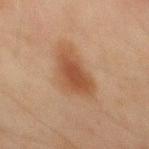| feature | finding |
|---|---|
| biopsy status | imaged on a skin check; not biopsied |
| body site | the mid back |
| diameter | ~6 mm (longest diameter) |
| patient | male, approximately 45 years of age |
| imaging modality | ~15 mm tile from a whole-body skin photo |